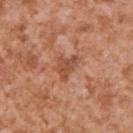Clinical impression:
The lesion was tiled from a total-body skin photograph and was not biopsied.
Image and clinical context:
A close-up tile cropped from a whole-body skin photograph, about 15 mm across. Captured under white-light illumination. Longest diameter approximately 3.5 mm. The patient is a male aged 43 to 47. The lesion is on the right upper arm.